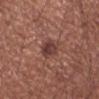Q: Is there a histopathology result?
A: imaged on a skin check; not biopsied
Q: Patient demographics?
A: male, aged approximately 60
Q: Where on the body is the lesion?
A: the left forearm
Q: How was the tile lit?
A: white-light illumination
Q: What is the lesion's diameter?
A: ≈3 mm
Q: What is the imaging modality?
A: 15 mm crop, total-body photography